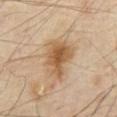Recorded during total-body skin imaging; not selected for excision or biopsy. Imaged with cross-polarized lighting. Located on the abdomen. Cropped from a whole-body photographic skin survey; the tile spans about 15 mm. A male subject about 70 years old.